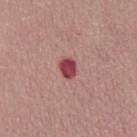Findings:
– anatomic site — the chest
– lesion size — ≈2.5 mm
– image — ~15 mm tile from a whole-body skin photo
– automated lesion analysis — an area of roughly 4.5 mm², an outline eccentricity of about 0.6 (0 = round, 1 = elongated), and a shape-asymmetry score of about 0.1 (0 = symmetric); a lesion color around L≈45 a*≈33 b*≈20 in CIELAB, about 16 CIELAB-L* units darker than the surrounding skin, and a normalized border contrast of about 11.5
– patient — female, about 35 years old